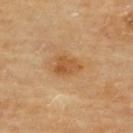Imaged during a routine full-body skin examination; the lesion was not biopsied and no histopathology is available.
Imaged with cross-polarized lighting.
A female subject aged around 70.
The lesion is on the upper back.
Longest diameter approximately 3.5 mm.
A region of skin cropped from a whole-body photographic capture, roughly 15 mm wide.